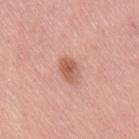Impression:
Part of a total-body skin-imaging series; this lesion was reviewed on a skin check and was not flagged for biopsy.
Acquisition and patient details:
From the right thigh. A female patient aged approximately 40. This is a white-light tile. A region of skin cropped from a whole-body photographic capture, roughly 15 mm wide.